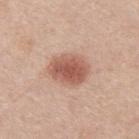| feature | finding |
|---|---|
| workup | total-body-photography surveillance lesion; no biopsy |
| subject | female, about 55 years old |
| lesion diameter | ≈4.5 mm |
| lighting | white-light illumination |
| body site | the upper back |
| automated lesion analysis | an outline eccentricity of about 0.65 (0 = round, 1 = elongated) and a symmetry-axis asymmetry near 0.1; border irregularity of about 1 on a 0–10 scale, a color-variation rating of about 4/10, and a peripheral color-asymmetry measure near 1 |
| image | 15 mm crop, total-body photography |